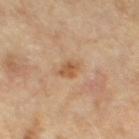Captured during whole-body skin photography for melanoma surveillance; the lesion was not biopsied. A female subject approximately 50 years of age. A roughly 15 mm field-of-view crop from a total-body skin photograph. This is a cross-polarized tile. On the right thigh. The lesion's longest dimension is about 2.5 mm.